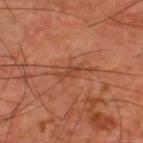Imaged during a routine full-body skin examination; the lesion was not biopsied and no histopathology is available. This image is a 15 mm lesion crop taken from a total-body photograph. Located on the upper back. A male subject aged 58–62.